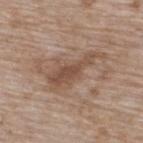Findings:
* workup: imaged on a skin check; not biopsied
* lighting: white-light
* anatomic site: the back
* image: ~15 mm tile from a whole-body skin photo
* subject: female, aged 73–77
* lesion diameter: ~6.5 mm (longest diameter)